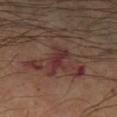| field | value |
|---|---|
| workup | catalogued during a skin exam; not biopsied |
| lesion size | ≈4 mm |
| lighting | cross-polarized |
| imaging modality | ~15 mm crop, total-body skin-cancer survey |
| location | the left lower leg |
| patient | male, in their 60s |
| automated metrics | a lesion area of about 5.5 mm², an eccentricity of roughly 0.9, and two-axis asymmetry of about 0.4; an average lesion color of about L≈30 a*≈21 b*≈18 (CIELAB), about 8 CIELAB-L* units darker than the surrounding skin, and a lesion-to-skin contrast of about 8 (normalized; higher = more distinct) |